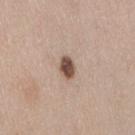acquisition: ~15 mm crop, total-body skin-cancer survey
patient: female, aged 38–42
anatomic site: the right thigh